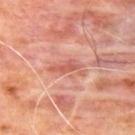This lesion was catalogued during total-body skin photography and was not selected for biopsy. Approximately 4 mm at its widest. Located on the upper back. Captured under cross-polarized illumination. A male subject, roughly 65 years of age. Cropped from a whole-body photographic skin survey; the tile spans about 15 mm. An algorithmic analysis of the crop reported a within-lesion color-variation index near 1.5/10. The analysis additionally found an automated nevus-likeness rating near 0 out of 100 and a detector confidence of about 60 out of 100 that the crop contains a lesion.